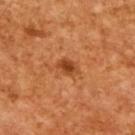workup — no biopsy performed (imaged during a skin exam) | diameter — ≈3 mm | image source — ~15 mm crop, total-body skin-cancer survey | subject — male, aged 63 to 67 | lighting — cross-polarized.